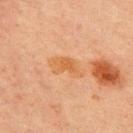biopsy status=imaged on a skin check; not biopsied
acquisition=total-body-photography crop, ~15 mm field of view
site=the chest
subject=male, about 70 years old
illumination=cross-polarized
automated metrics=a lesion color around L≈51 a*≈21 b*≈37 in CIELAB and roughly 6 lightness units darker than nearby skin; a color-variation rating of about 2.5/10 and a peripheral color-asymmetry measure near 0.5